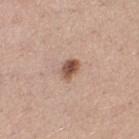{
  "biopsy_status": "not biopsied; imaged during a skin examination",
  "patient": {
    "sex": "female",
    "age_approx": 45
  },
  "site": "left thigh",
  "image": {
    "source": "total-body photography crop",
    "field_of_view_mm": 15
  }
}